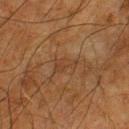biopsy status: total-body-photography surveillance lesion; no biopsy | patient: male, aged around 65 | body site: the right lower leg | image source: total-body-photography crop, ~15 mm field of view | illumination: cross-polarized illumination.